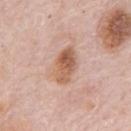Captured during whole-body skin photography for melanoma surveillance; the lesion was not biopsied.
The subject is a male in their 80s.
The lesion is located on the chest.
Captured under white-light illumination.
A 15 mm crop from a total-body photograph taken for skin-cancer surveillance.
Longest diameter approximately 5 mm.